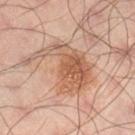Part of a total-body skin-imaging series; this lesion was reviewed on a skin check and was not flagged for biopsy. The lesion is on the right lower leg. The recorded lesion diameter is about 7.5 mm. A male patient aged around 40. This is a cross-polarized tile. A region of skin cropped from a whole-body photographic capture, roughly 15 mm wide.